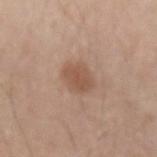<record>
  <biopsy_status>not biopsied; imaged during a skin examination</biopsy_status>
  <image>
    <source>total-body photography crop</source>
    <field_of_view_mm>15</field_of_view_mm>
  </image>
  <lighting>white-light</lighting>
  <site>left forearm</site>
  <patient>
    <sex>male</sex>
    <age_approx>60</age_approx>
  </patient>
  <automated_metrics>
    <area_mm2_approx>6.5</area_mm2_approx>
    <eccentricity>0.6</eccentricity>
    <shape_asymmetry>0.15</shape_asymmetry>
    <border_irregularity_0_10>1.5</border_irregularity_0_10>
    <color_variation_0_10>2.0</color_variation_0_10>
    <peripheral_color_asymmetry>0.5</peripheral_color_asymmetry>
    <nevus_likeness_0_100>65</nevus_likeness_0_100>
    <lesion_detection_confidence_0_100>100</lesion_detection_confidence_0_100>
  </automated_metrics>
  <lesion_size>
    <long_diameter_mm_approx>3.0</long_diameter_mm_approx>
  </lesion_size>
</record>